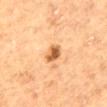Assessment: Imaged during a routine full-body skin examination; the lesion was not biopsied and no histopathology is available. Acquisition and patient details: A 15 mm close-up tile from a total-body photography series done for melanoma screening. Imaged with cross-polarized lighting. The subject is a female about 60 years old. The lesion's longest dimension is about 3 mm. On the mid back.